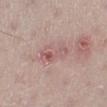| key | value |
|---|---|
| notes | total-body-photography surveillance lesion; no biopsy |
| lesion size | ≈4.5 mm |
| automated lesion analysis | a lesion area of about 7 mm², an eccentricity of roughly 0.9, and a shape-asymmetry score of about 0.4 (0 = symmetric); a lesion color around L≈57 a*≈22 b*≈21 in CIELAB, about 8 CIELAB-L* units darker than the surrounding skin, and a normalized border contrast of about 5.5; a classifier nevus-likeness of about 0/100 and a lesion-detection confidence of about 100/100 |
| acquisition | 15 mm crop, total-body photography |
| subject | male, about 60 years old |
| anatomic site | the left lower leg |